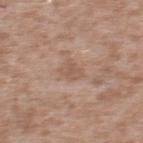Part of a total-body skin-imaging series; this lesion was reviewed on a skin check and was not flagged for biopsy. Approximately 2.5 mm at its widest. Captured under white-light illumination. The patient is a male aged around 45. From the upper back. A close-up tile cropped from a whole-body skin photograph, about 15 mm across.